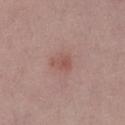workup: total-body-photography surveillance lesion; no biopsy | site: the left lower leg | tile lighting: white-light illumination | image-analysis metrics: an area of roughly 4 mm², an outline eccentricity of about 0.8 (0 = round, 1 = elongated), and a symmetry-axis asymmetry near 0.3; about 7 CIELAB-L* units darker than the surrounding skin and a normalized border contrast of about 5.5; internal color variation of about 2 on a 0–10 scale and radial color variation of about 0.5; a lesion-detection confidence of about 100/100 | image: ~15 mm crop, total-body skin-cancer survey | size: ~2.5 mm (longest diameter) | subject: female, aged 48–52.